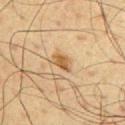Impression: Imaged during a routine full-body skin examination; the lesion was not biopsied and no histopathology is available. Background: From the chest. This image is a 15 mm lesion crop taken from a total-body photograph. The tile uses cross-polarized illumination. Approximately 3 mm at its widest. The patient is a male about 60 years old.